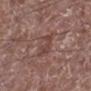Q: Was this lesion biopsied?
A: catalogued during a skin exam; not biopsied